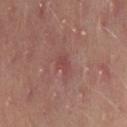lighting: cross-polarized illumination; lesion size: ≈2.5 mm; location: the right thigh; patient: female, aged approximately 50; image source: total-body-photography crop, ~15 mm field of view.